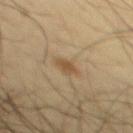Impression: No biopsy was performed on this lesion — it was imaged during a full skin examination and was not determined to be concerning. Background: The recorded lesion diameter is about 2 mm. An algorithmic analysis of the crop reported a nevus-likeness score of about 75/100 and a detector confidence of about 100 out of 100 that the crop contains a lesion. A 15 mm crop from a total-body photograph taken for skin-cancer surveillance. A male patient in their mid- to late 40s. Located on the mid back. The tile uses cross-polarized illumination.